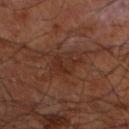Assessment: Captured during whole-body skin photography for melanoma surveillance; the lesion was not biopsied. Image and clinical context: Measured at roughly 3.5 mm in maximum diameter. An algorithmic analysis of the crop reported a lesion color around L≈28 a*≈20 b*≈25 in CIELAB, roughly 5 lightness units darker than nearby skin, and a lesion-to-skin contrast of about 5.5 (normalized; higher = more distinct). Captured under cross-polarized illumination. A lesion tile, about 15 mm wide, cut from a 3D total-body photograph. The subject is a male aged approximately 60. From the leg.